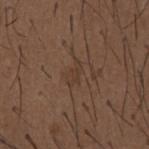Notes:
• notes: no biopsy performed (imaged during a skin exam)